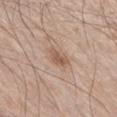Captured during whole-body skin photography for melanoma surveillance; the lesion was not biopsied.